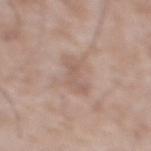Imaged during a routine full-body skin examination; the lesion was not biopsied and no histopathology is available. Longest diameter approximately 4.5 mm. A lesion tile, about 15 mm wide, cut from a 3D total-body photograph. The lesion is located on the mid back. A male subject, about 50 years old. Imaged with white-light lighting.